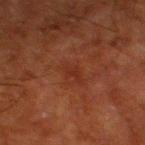| field | value |
|---|---|
| workup | imaged on a skin check; not biopsied |
| illumination | cross-polarized illumination |
| patient | male, approximately 80 years of age |
| automated lesion analysis | an area of roughly 4 mm², an outline eccentricity of about 0.9 (0 = round, 1 = elongated), and two-axis asymmetry of about 0.35; a color-variation rating of about 0.5/10 and a peripheral color-asymmetry measure near 0 |
| size | about 3.5 mm |
| acquisition | 15 mm crop, total-body photography |
| anatomic site | the leg |